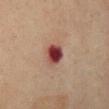This lesion was catalogued during total-body skin photography and was not selected for biopsy. The recorded lesion diameter is about 3 mm. From the chest. The patient is a female roughly 70 years of age. A lesion tile, about 15 mm wide, cut from a 3D total-body photograph. The total-body-photography lesion software estimated a footprint of about 6 mm² and a shape-asymmetry score of about 0.15 (0 = symmetric). And it measured a lesion color around L≈36 a*≈25 b*≈21 in CIELAB and a normalized border contrast of about 13.5. Captured under cross-polarized illumination.